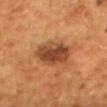Imaged during a routine full-body skin examination; the lesion was not biopsied and no histopathology is available.
From the mid back.
A female patient, about 50 years old.
The recorded lesion diameter is about 5.5 mm.
The lesion-visualizer software estimated an area of roughly 13 mm², a shape eccentricity near 0.75, and a shape-asymmetry score of about 0.2 (0 = symmetric). The analysis additionally found a border-irregularity rating of about 2/10, internal color variation of about 4.5 on a 0–10 scale, and radial color variation of about 1.5. It also reported a nevus-likeness score of about 75/100 and lesion-presence confidence of about 100/100.
Cropped from a total-body skin-imaging series; the visible field is about 15 mm.